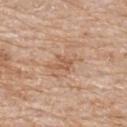Impression: Captured during whole-body skin photography for melanoma surveillance; the lesion was not biopsied. Context: A 15 mm close-up tile from a total-body photography series done for melanoma screening. The lesion is on the upper back. Imaged with white-light lighting. Measured at roughly 2.5 mm in maximum diameter. Automated tile analysis of the lesion measured an automated nevus-likeness rating near 0 out of 100. A female subject aged 83 or older.